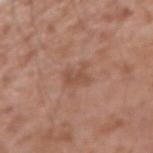Imaged during a routine full-body skin examination; the lesion was not biopsied and no histopathology is available. A close-up tile cropped from a whole-body skin photograph, about 15 mm across. A male patient, approximately 75 years of age. Longest diameter approximately 3 mm. Located on the right forearm.